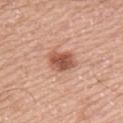| field | value |
|---|---|
| notes | catalogued during a skin exam; not biopsied |
| image source | ~15 mm tile from a whole-body skin photo |
| automated metrics | a nevus-likeness score of about 95/100 and lesion-presence confidence of about 100/100 |
| illumination | white-light illumination |
| subject | male, in their mid-60s |
| site | the left upper arm |